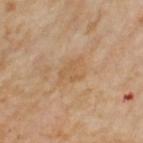No biopsy was performed on this lesion — it was imaged during a full skin examination and was not determined to be concerning. The lesion is on the left upper arm. Imaged with cross-polarized lighting. Automated tile analysis of the lesion measured a lesion area of about 5.5 mm² and a shape-asymmetry score of about 0.45 (0 = symmetric). The analysis additionally found a border-irregularity index near 5.5/10 and radial color variation of about 0.5. A roughly 15 mm field-of-view crop from a total-body skin photograph. Longest diameter approximately 4 mm. The subject is a female about 55 years old.